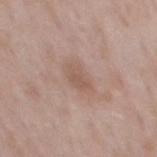Findings:
* notes: imaged on a skin check; not biopsied
* anatomic site: the mid back
* lesion diameter: ≈3 mm
* subject: male, aged approximately 55
* acquisition: 15 mm crop, total-body photography
* tile lighting: white-light